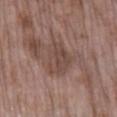Acquisition and patient details: Longest diameter approximately 4.5 mm. The lesion is on the right lower leg. The patient is a female approximately 75 years of age. Captured under white-light illumination. Automated image analysis of the tile measured border irregularity of about 3.5 on a 0–10 scale and peripheral color asymmetry of about 1. The software also gave an automated nevus-likeness rating near 0 out of 100. This image is a 15 mm lesion crop taken from a total-body photograph.